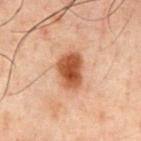{
  "biopsy_status": "not biopsied; imaged during a skin examination",
  "lighting": "cross-polarized",
  "lesion_size": {
    "long_diameter_mm_approx": 4.0
  },
  "automated_metrics": {
    "border_irregularity_0_10": 2.5,
    "color_variation_0_10": 3.5,
    "peripheral_color_asymmetry": 1.0
  },
  "patient": {
    "sex": "male",
    "age_approx": 60
  },
  "image": {
    "source": "total-body photography crop",
    "field_of_view_mm": 15
  },
  "site": "chest"
}